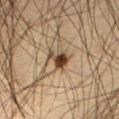Notes:
• workup · no biopsy performed (imaged during a skin exam)
• subject · male, aged around 60
• body site · the abdomen
• automated metrics · an area of roughly 4.5 mm², a shape eccentricity near 0.65, and a shape-asymmetry score of about 0.5 (0 = symmetric); a mean CIELAB color near L≈35 a*≈14 b*≈26, about 15 CIELAB-L* units darker than the surrounding skin, and a normalized border contrast of about 13
• acquisition · total-body-photography crop, ~15 mm field of view
• diameter · ~2.5 mm (longest diameter)